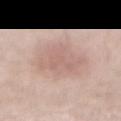No biopsy was performed on this lesion — it was imaged during a full skin examination and was not determined to be concerning. The tile uses white-light illumination. The lesion-visualizer software estimated a classifier nevus-likeness of about 0/100 and lesion-presence confidence of about 100/100. A male subject about 70 years old. The lesion is on the abdomen. This image is a 15 mm lesion crop taken from a total-body photograph. The recorded lesion diameter is about 6 mm.